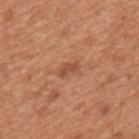Image and clinical context: On the arm. The subject is a male aged 63–67. Cropped from a total-body skin-imaging series; the visible field is about 15 mm.